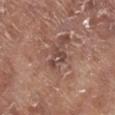Q: Was a biopsy performed?
A: total-body-photography surveillance lesion; no biopsy
Q: Automated lesion metrics?
A: a border-irregularity index near 5.5/10, a color-variation rating of about 4.5/10, and radial color variation of about 2; a nevus-likeness score of about 0/100 and a detector confidence of about 50 out of 100 that the crop contains a lesion
Q: Patient demographics?
A: male, roughly 75 years of age
Q: Lesion size?
A: ≈3 mm
Q: How was this image acquired?
A: ~15 mm crop, total-body skin-cancer survey
Q: How was the tile lit?
A: white-light
Q: What is the anatomic site?
A: the leg